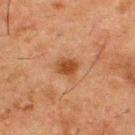follow-up — imaged on a skin check; not biopsied | image — total-body-photography crop, ~15 mm field of view | patient — male, approximately 50 years of age | anatomic site — the upper back | illumination — cross-polarized.